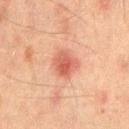Findings:
• follow-up: imaged on a skin check; not biopsied
• lesion diameter: ~3 mm (longest diameter)
• subject: male, approximately 45 years of age
• acquisition: ~15 mm tile from a whole-body skin photo
• location: the chest
• tile lighting: cross-polarized illumination